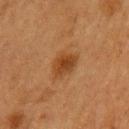Part of a total-body skin-imaging series; this lesion was reviewed on a skin check and was not flagged for biopsy. The total-body-photography lesion software estimated a footprint of about 6.5 mm² and an eccentricity of roughly 0.6. And it measured border irregularity of about 2.5 on a 0–10 scale and a peripheral color-asymmetry measure near 1. Captured under cross-polarized illumination. Located on the left upper arm. Cropped from a whole-body photographic skin survey; the tile spans about 15 mm. The patient is a female aged approximately 55. Longest diameter approximately 3 mm.